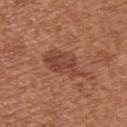Findings:
* anatomic site: the back
* patient: female, in their 40s
* image source: 15 mm crop, total-body photography